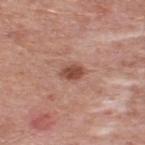Imaged with white-light lighting.
Longest diameter approximately 2.5 mm.
A male patient in their mid-50s.
An algorithmic analysis of the crop reported a within-lesion color-variation index near 2.5/10 and peripheral color asymmetry of about 1. The software also gave an automated nevus-likeness rating near 85 out of 100 and lesion-presence confidence of about 100/100.
On the upper back.
A roughly 15 mm field-of-view crop from a total-body skin photograph.